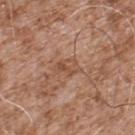biopsy status — no biopsy performed (imaged during a skin exam)
lesion size — about 3 mm
patient — male, about 55 years old
TBP lesion metrics — a footprint of about 4.5 mm², an outline eccentricity of about 0.8 (0 = round, 1 = elongated), and a shape-asymmetry score of about 0.35 (0 = symmetric); border irregularity of about 3.5 on a 0–10 scale and peripheral color asymmetry of about 2
anatomic site — the back
image — ~15 mm crop, total-body skin-cancer survey